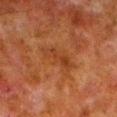Assessment: No biopsy was performed on this lesion — it was imaged during a full skin examination and was not determined to be concerning. Acquisition and patient details: A male subject, aged approximately 80. On the left lower leg. Approximately 5.5 mm at its widest. Cropped from a whole-body photographic skin survey; the tile spans about 15 mm. Imaged with cross-polarized lighting.